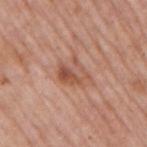Imaged during a routine full-body skin examination; the lesion was not biopsied and no histopathology is available. The total-body-photography lesion software estimated an area of roughly 7 mm² and two-axis asymmetry of about 0.35. And it measured radial color variation of about 3.5. It also reported a classifier nevus-likeness of about 0/100 and a detector confidence of about 100 out of 100 that the crop contains a lesion. A lesion tile, about 15 mm wide, cut from a 3D total-body photograph. The subject is a male aged around 75. Captured under white-light illumination. Located on the right upper arm.